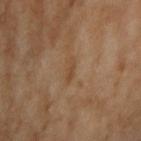Captured during whole-body skin photography for melanoma surveillance; the lesion was not biopsied. A female subject aged around 60. Approximately 3 mm at its widest. A region of skin cropped from a whole-body photographic capture, roughly 15 mm wide. The lesion is located on the right upper arm. Automated tile analysis of the lesion measured border irregularity of about 4 on a 0–10 scale and a peripheral color-asymmetry measure near 0. This is a cross-polarized tile.